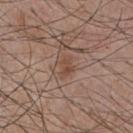biopsy_status: not biopsied; imaged during a skin examination
image:
  source: total-body photography crop
  field_of_view_mm: 15
patient:
  sex: male
  age_approx: 55
lesion_size:
  long_diameter_mm_approx: 3.0
site: chest
lighting: white-light